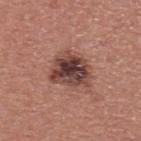biopsy status — imaged on a skin check; not biopsied
anatomic site — the upper back
lighting — white-light illumination
imaging modality — total-body-photography crop, ~15 mm field of view
lesion diameter — ≈5 mm
patient — male, aged around 40